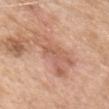• anatomic site · the front of the torso
• subject · female, aged around 70
• image source · ~15 mm tile from a whole-body skin photo